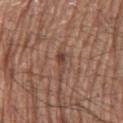Part of a total-body skin-imaging series; this lesion was reviewed on a skin check and was not flagged for biopsy. A lesion tile, about 15 mm wide, cut from a 3D total-body photograph. The lesion is located on the left thigh. Automated tile analysis of the lesion measured a lesion area of about 2.5 mm², an outline eccentricity of about 0.95 (0 = round, 1 = elongated), and a symmetry-axis asymmetry near 0.4. The analysis additionally found a lesion color around L≈43 a*≈20 b*≈26 in CIELAB and a normalized lesion–skin contrast near 7. It also reported a nevus-likeness score of about 5/100 and lesion-presence confidence of about 95/100. A male patient aged around 70. The tile uses white-light illumination.